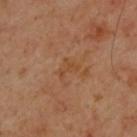workup = total-body-photography surveillance lesion; no biopsy | size = ~2.5 mm (longest diameter) | subject = male, aged 43–47 | body site = the upper back | image = ~15 mm crop, total-body skin-cancer survey | illumination = cross-polarized illumination.